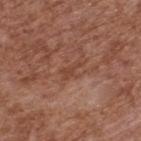| feature | finding |
|---|---|
| biopsy status | imaged on a skin check; not biopsied |
| location | the upper back |
| image-analysis metrics | a border-irregularity index near 5/10, a within-lesion color-variation index near 0.5/10, and peripheral color asymmetry of about 0 |
| patient | male, aged approximately 75 |
| illumination | white-light illumination |
| image source | 15 mm crop, total-body photography |
| lesion size | about 2.5 mm |